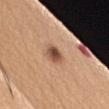Recorded during total-body skin imaging; not selected for excision or biopsy. The recorded lesion diameter is about 2.5 mm. A female subject, aged 58–62. Automated tile analysis of the lesion measured roughly 15 lightness units darker than nearby skin and a lesion-to-skin contrast of about 10 (normalized; higher = more distinct). And it measured a border-irregularity index near 2/10, a color-variation rating of about 5/10, and radial color variation of about 1.5. The software also gave a classifier nevus-likeness of about 95/100 and lesion-presence confidence of about 100/100. A close-up tile cropped from a whole-body skin photograph, about 15 mm across. This is a white-light tile. Located on the left upper arm.